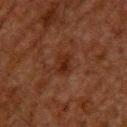biopsy status: imaged on a skin check; not biopsied | diameter: about 3 mm | body site: the upper back | lighting: cross-polarized illumination | patient: male, roughly 60 years of age | image source: ~15 mm tile from a whole-body skin photo.